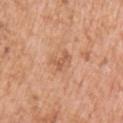Impression: Imaged during a routine full-body skin examination; the lesion was not biopsied and no histopathology is available. Context: Longest diameter approximately 2.5 mm. A lesion tile, about 15 mm wide, cut from a 3D total-body photograph. A female patient aged approximately 40. An algorithmic analysis of the crop reported a lesion color around L≈58 a*≈24 b*≈34 in CIELAB, a lesion–skin lightness drop of about 9, and a lesion-to-skin contrast of about 6 (normalized; higher = more distinct). The analysis additionally found border irregularity of about 5.5 on a 0–10 scale, a within-lesion color-variation index near 0/10, and peripheral color asymmetry of about 0. The analysis additionally found a nevus-likeness score of about 0/100 and lesion-presence confidence of about 100/100. The lesion is on the left upper arm.